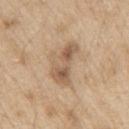Part of a total-body skin-imaging series; this lesion was reviewed on a skin check and was not flagged for biopsy.
Approximately 5 mm at its widest.
The tile uses white-light illumination.
A male patient aged around 70.
On the left upper arm.
This image is a 15 mm lesion crop taken from a total-body photograph.